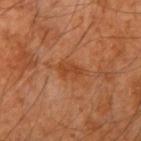Recorded during total-body skin imaging; not selected for excision or biopsy.
Captured under cross-polarized illumination.
About 3 mm across.
A region of skin cropped from a whole-body photographic capture, roughly 15 mm wide.
A male patient aged 58 to 62.
The lesion is located on the right upper arm.